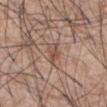Captured during whole-body skin photography for melanoma surveillance; the lesion was not biopsied. Longest diameter approximately 3 mm. Cropped from a total-body skin-imaging series; the visible field is about 15 mm. The patient is a male in their 60s. The lesion is located on the abdomen. Captured under white-light illumination. The total-body-photography lesion software estimated a lesion area of about 4.5 mm², a shape eccentricity near 0.8, and a symmetry-axis asymmetry near 0.35. The analysis additionally found about 9 CIELAB-L* units darker than the surrounding skin and a normalized lesion–skin contrast near 6.5. It also reported internal color variation of about 4.5 on a 0–10 scale and peripheral color asymmetry of about 1.5.